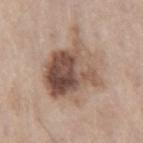{
  "diagnosis": {
    "histopathology": "invasive melanoma, superficial spreading type",
    "malignancy": "malignant",
    "taxonomic_path": [
      "Malignant",
      "Malignant melanocytic proliferations (Melanoma)",
      "Melanoma Invasive",
      "Melanoma Invasive, Superficial spreading"
    ],
    "breslow_mm": 0.2,
    "mitotic_index": "<1/mm²"
  }
}